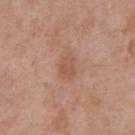Recorded during total-body skin imaging; not selected for excision or biopsy. A male subject roughly 70 years of age. The tile uses white-light illumination. Located on the abdomen. The lesion-visualizer software estimated an area of roughly 4.5 mm², a shape eccentricity near 0.7, and a shape-asymmetry score of about 0.3 (0 = symmetric). The software also gave a mean CIELAB color near L≈54 a*≈22 b*≈30, about 7 CIELAB-L* units darker than the surrounding skin, and a lesion-to-skin contrast of about 5.5 (normalized; higher = more distinct). The analysis additionally found a border-irregularity index near 2.5/10 and a within-lesion color-variation index near 2.5/10. And it measured an automated nevus-likeness rating near 0 out of 100 and a lesion-detection confidence of about 100/100. A roughly 15 mm field-of-view crop from a total-body skin photograph. The lesion's longest dimension is about 2.5 mm.